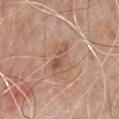Findings:
- biopsy status — total-body-photography surveillance lesion; no biopsy
- image — total-body-photography crop, ~15 mm field of view
- patient — male, roughly 80 years of age
- body site — the chest